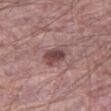workup=imaged on a skin check; not biopsied | illumination=white-light | size=≈3.5 mm | image source=total-body-photography crop, ~15 mm field of view | subject=male, about 70 years old | location=the right thigh | automated lesion analysis=a lesion color around L≈46 a*≈21 b*≈19 in CIELAB and roughly 12 lightness units darker than nearby skin.